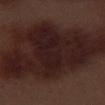<case>
<biopsy_status>not biopsied; imaged during a skin examination</biopsy_status>
<lesion_size>
  <long_diameter_mm_approx>17.0</long_diameter_mm_approx>
</lesion_size>
<patient>
  <sex>male</sex>
  <age_approx>70</age_approx>
</patient>
<site>right thigh</site>
<lighting>white-light</lighting>
<image>
  <source>total-body photography crop</source>
  <field_of_view_mm>15</field_of_view_mm>
</image>
<automated_metrics>
  <eccentricity>0.9</eccentricity>
  <shape_asymmetry>0.4</shape_asymmetry>
  <border_irregularity_0_10>8.0</border_irregularity_0_10>
  <color_variation_0_10>3.5</color_variation_0_10>
  <peripheral_color_asymmetry>1.0</peripheral_color_asymmetry>
</automated_metrics>
</case>